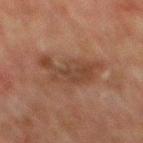{"site": "mid back", "patient": {"sex": "male", "age_approx": 60}, "lighting": "cross-polarized", "lesion_size": {"long_diameter_mm_approx": 5.5}, "automated_metrics": {"area_mm2_approx": 13.0, "eccentricity": 0.75, "shape_asymmetry": 0.5, "border_irregularity_0_10": 6.5, "color_variation_0_10": 2.5, "nevus_likeness_0_100": 0}, "image": {"source": "total-body photography crop", "field_of_view_mm": 15}}